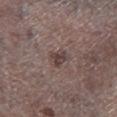The lesion is located on the leg. The total-body-photography lesion software estimated a mean CIELAB color near L≈41 a*≈14 b*≈17, roughly 8 lightness units darker than nearby skin, and a normalized lesion–skin contrast near 7. It also reported a classifier nevus-likeness of about 0/100 and a detector confidence of about 100 out of 100 that the crop contains a lesion. A male subject, about 70 years old. The lesion's longest dimension is about 3 mm. A 15 mm close-up extracted from a 3D total-body photography capture.